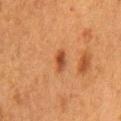| feature | finding |
|---|---|
| notes | catalogued during a skin exam; not biopsied |
| body site | the chest |
| subject | female, about 50 years old |
| image source | ~15 mm crop, total-body skin-cancer survey |